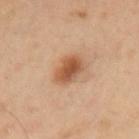Background:
The recorded lesion diameter is about 3.5 mm. Located on the left upper arm. The total-body-photography lesion software estimated an area of roughly 7 mm², an eccentricity of roughly 0.7, and a symmetry-axis asymmetry near 0.15. The analysis additionally found an automated nevus-likeness rating near 100 out of 100 and a lesion-detection confidence of about 100/100. A male patient aged approximately 40. A lesion tile, about 15 mm wide, cut from a 3D total-body photograph. The tile uses cross-polarized illumination.